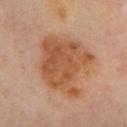The lesion was tiled from a total-body skin photograph and was not biopsied. The patient is a female aged 58 to 62. Imaged with cross-polarized lighting. This image is a 15 mm lesion crop taken from a total-body photograph. Located on the chest. Automated tile analysis of the lesion measured a lesion color around L≈55 a*≈23 b*≈36 in CIELAB and roughly 10 lightness units darker than nearby skin. The analysis additionally found a nevus-likeness score of about 20/100 and a detector confidence of about 100 out of 100 that the crop contains a lesion.